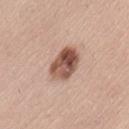This image is a 15 mm lesion crop taken from a total-body photograph. A female patient aged around 40. The lesion is on the left thigh. Captured under white-light illumination. The recorded lesion diameter is about 4.5 mm. The total-body-photography lesion software estimated an outline eccentricity of about 0.7 (0 = round, 1 = elongated) and a symmetry-axis asymmetry near 0.2. The analysis additionally found a border-irregularity rating of about 1.5/10 and a peripheral color-asymmetry measure near 4. It also reported a classifier nevus-likeness of about 60/100 and lesion-presence confidence of about 100/100.